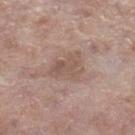This lesion was catalogued during total-body skin photography and was not selected for biopsy. The patient is a female approximately 85 years of age or older. The lesion is located on the right lower leg. A close-up tile cropped from a whole-body skin photograph, about 15 mm across. The tile uses white-light illumination.